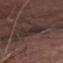size: ~4.5 mm (longest diameter) | tile lighting: white-light illumination | patient: male, in their mid- to late 70s | image source: 15 mm crop, total-body photography | anatomic site: the abdomen | TBP lesion metrics: an area of roughly 9.5 mm² and a shape eccentricity near 0.55; border irregularity of about 5 on a 0–10 scale, a within-lesion color-variation index near 2.5/10, and a peripheral color-asymmetry measure near 0.5; an automated nevus-likeness rating near 0 out of 100 and a lesion-detection confidence of about 65/100.